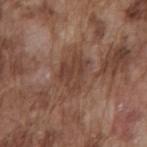Clinical impression:
Captured during whole-body skin photography for melanoma surveillance; the lesion was not biopsied.
Image and clinical context:
Cropped from a whole-body photographic skin survey; the tile spans about 15 mm. Located on the mid back. Automated tile analysis of the lesion measured an average lesion color of about L≈41 a*≈19 b*≈26 (CIELAB), about 8 CIELAB-L* units darker than the surrounding skin, and a normalized lesion–skin contrast near 7. Captured under white-light illumination. Measured at roughly 4 mm in maximum diameter. A male subject roughly 75 years of age.